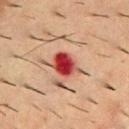follow-up: catalogued during a skin exam; not biopsied.